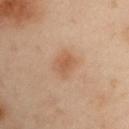The lesion was tiled from a total-body skin photograph and was not biopsied. The patient is a male about 55 years old. The lesion's longest dimension is about 3 mm. The lesion-visualizer software estimated an average lesion color of about L≈46 a*≈16 b*≈28 (CIELAB), about 6 CIELAB-L* units darker than the surrounding skin, and a normalized lesion–skin contrast near 6. The analysis additionally found a nevus-likeness score of about 65/100 and a detector confidence of about 100 out of 100 that the crop contains a lesion. The lesion is on the right upper arm. Captured under cross-polarized illumination. Cropped from a whole-body photographic skin survey; the tile spans about 15 mm.